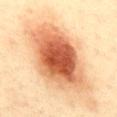Part of a total-body skin-imaging series; this lesion was reviewed on a skin check and was not flagged for biopsy. A 15 mm crop from a total-body photograph taken for skin-cancer surveillance. A female subject aged around 45. Located on the back.